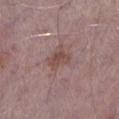Measured at roughly 3 mm in maximum diameter.
Captured under white-light illumination.
A male subject, in their mid-70s.
The lesion is located on the left lower leg.
Cropped from a whole-body photographic skin survey; the tile spans about 15 mm.